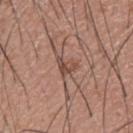  biopsy_status: not biopsied; imaged during a skin examination